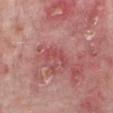The lesion was tiled from a total-body skin photograph and was not biopsied.
Located on the arm.
Captured under white-light illumination.
A 15 mm crop from a total-body photograph taken for skin-cancer surveillance.
A male subject roughly 75 years of age.
An algorithmic analysis of the crop reported a lesion area of about 4 mm², an outline eccentricity of about 0.95 (0 = round, 1 = elongated), and a symmetry-axis asymmetry near 0.55. The software also gave a lesion color around L≈50 a*≈32 b*≈23 in CIELAB, a lesion–skin lightness drop of about 7, and a normalized border contrast of about 5. And it measured a border-irregularity rating of about 7.5/10, a within-lesion color-variation index near 0/10, and a peripheral color-asymmetry measure near 0.
The recorded lesion diameter is about 3.5 mm.